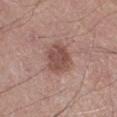  biopsy_status: not biopsied; imaged during a skin examination
  lesion_size:
    long_diameter_mm_approx: 3.5
  lighting: white-light
  patient:
    sex: male
    age_approx: 65
  site: right lower leg
  image:
    source: total-body photography crop
    field_of_view_mm: 15